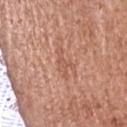The recorded lesion diameter is about 4 mm.
From the head or neck.
A lesion tile, about 15 mm wide, cut from a 3D total-body photograph.
The patient is a female aged around 35.